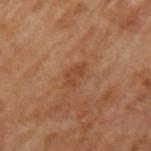{"biopsy_status": "not biopsied; imaged during a skin examination", "automated_metrics": {"area_mm2_approx": 4.5, "eccentricity": 0.85, "shape_asymmetry": 0.35}, "image": {"source": "total-body photography crop", "field_of_view_mm": 15}, "lesion_size": {"long_diameter_mm_approx": 3.5}, "lighting": "cross-polarized", "patient": {"sex": "male", "age_approx": 65}}